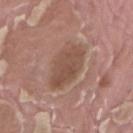<lesion>
<biopsy_status>not biopsied; imaged during a skin examination</biopsy_status>
<site>right thigh</site>
<patient>
  <sex>male</sex>
  <age_approx>40</age_approx>
</patient>
<image>
  <source>total-body photography crop</source>
  <field_of_view_mm>15</field_of_view_mm>
</image>
</lesion>